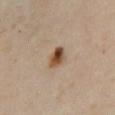| feature | finding |
|---|---|
| follow-up | imaged on a skin check; not biopsied |
| imaging modality | ~15 mm crop, total-body skin-cancer survey |
| lighting | cross-polarized |
| lesion size | ~3 mm (longest diameter) |
| anatomic site | the front of the torso |
| subject | male, roughly 65 years of age |